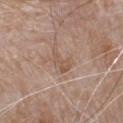Case summary:
* notes — catalogued during a skin exam; not biopsied
* automated lesion analysis — a lesion area of about 5.5 mm², a shape eccentricity near 0.8, and a shape-asymmetry score of about 0.4 (0 = symmetric); a lesion color around L≈55 a*≈17 b*≈28 in CIELAB, a lesion–skin lightness drop of about 6, and a lesion-to-skin contrast of about 5 (normalized; higher = more distinct)
* site — the chest
* patient — male, about 80 years old
* lighting — white-light
* acquisition — total-body-photography crop, ~15 mm field of view